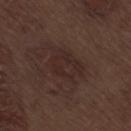This lesion was catalogued during total-body skin photography and was not selected for biopsy. A male subject, about 70 years old. A 15 mm close-up tile from a total-body photography series done for melanoma screening. Imaged with white-light lighting. About 5 mm across. The lesion is on the right thigh.